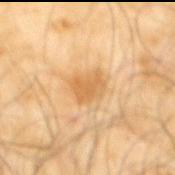notes: total-body-photography surveillance lesion; no biopsy
lesion diameter: ≈3 mm
location: the mid back
tile lighting: cross-polarized
imaging modality: ~15 mm crop, total-body skin-cancer survey
subject: male, roughly 65 years of age